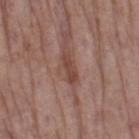Captured during whole-body skin photography for melanoma surveillance; the lesion was not biopsied.
A 15 mm close-up extracted from a 3D total-body photography capture.
The lesion is on the right thigh.
A female patient approximately 85 years of age or older.